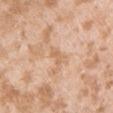Clinical impression:
The lesion was tiled from a total-body skin photograph and was not biopsied.